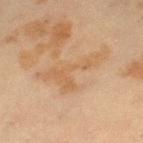Imaged during a routine full-body skin examination; the lesion was not biopsied and no histopathology is available.